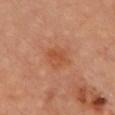Part of a total-body skin-imaging series; this lesion was reviewed on a skin check and was not flagged for biopsy. A male subject aged around 65. Cropped from a whole-body photographic skin survey; the tile spans about 15 mm. The lesion is located on the chest.